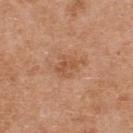biopsy status: catalogued during a skin exam; not biopsied | image: ~15 mm crop, total-body skin-cancer survey | patient: female, roughly 40 years of age | anatomic site: the back | image-analysis metrics: a mean CIELAB color near L≈54 a*≈24 b*≈35, roughly 8 lightness units darker than nearby skin, and a normalized border contrast of about 5.5; border irregularity of about 4.5 on a 0–10 scale and a color-variation rating of about 2/10.